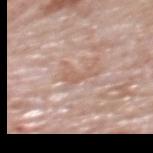– notes: catalogued during a skin exam; not biopsied